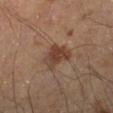Assessment: Captured during whole-body skin photography for melanoma surveillance; the lesion was not biopsied. Acquisition and patient details: On the right lower leg. A male patient aged 53 to 57. A 15 mm close-up tile from a total-body photography series done for melanoma screening.